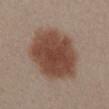Clinical impression: Part of a total-body skin-imaging series; this lesion was reviewed on a skin check and was not flagged for biopsy. Image and clinical context: From the mid back. Automated image analysis of the tile measured a footprint of about 36 mm², an eccentricity of roughly 0.7, and two-axis asymmetry of about 0.15. The analysis additionally found a lesion color around L≈45 a*≈19 b*≈26 in CIELAB, about 13 CIELAB-L* units darker than the surrounding skin, and a normalized border contrast of about 10.5. The software also gave a border-irregularity rating of about 1.5/10, internal color variation of about 4 on a 0–10 scale, and peripheral color asymmetry of about 1. The lesion's longest dimension is about 8 mm. A female subject aged 28 to 32. A roughly 15 mm field-of-view crop from a total-body skin photograph. This is a white-light tile.